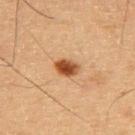Q: Was a biopsy performed?
A: no biopsy performed (imaged during a skin exam)
Q: What is the imaging modality?
A: 15 mm crop, total-body photography
Q: What did automated image analysis measure?
A: an area of roughly 5 mm², an outline eccentricity of about 0.7 (0 = round, 1 = elongated), and two-axis asymmetry of about 0.25; a border-irregularity rating of about 2.5/10, a color-variation rating of about 4.5/10, and radial color variation of about 1.5; an automated nevus-likeness rating near 100 out of 100 and a detector confidence of about 100 out of 100 that the crop contains a lesion
Q: Lesion size?
A: about 3 mm
Q: Patient demographics?
A: male, aged approximately 60